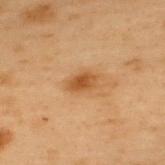Imaged with cross-polarized lighting. The lesion's longest dimension is about 3 mm. A roughly 15 mm field-of-view crop from a total-body skin photograph. The subject is a male aged 53–57. The lesion is located on the upper back. The total-body-photography lesion software estimated an outline eccentricity of about 0.8 (0 = round, 1 = elongated) and a shape-asymmetry score of about 0.25 (0 = symmetric). And it measured an average lesion color of about L≈43 a*≈20 b*≈35 (CIELAB), roughly 9 lightness units darker than nearby skin, and a normalized border contrast of about 8.